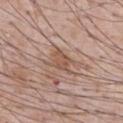biopsy_status: not biopsied; imaged during a skin examination
lighting: white-light
image:
  source: total-body photography crop
  field_of_view_mm: 15
patient:
  sex: male
  age_approx: 70
site: abdomen